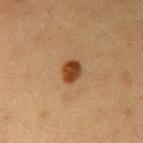Recorded during total-body skin imaging; not selected for excision or biopsy. The recorded lesion diameter is about 2.5 mm. Located on the right upper arm. The total-body-photography lesion software estimated an eccentricity of roughly 0.65 and a symmetry-axis asymmetry near 0.2. The analysis additionally found a mean CIELAB color near L≈34 a*≈20 b*≈32 and a normalized border contrast of about 11.5. It also reported a nevus-likeness score of about 100/100 and a detector confidence of about 100 out of 100 that the crop contains a lesion. The patient is a male about 40 years old. A lesion tile, about 15 mm wide, cut from a 3D total-body photograph. Captured under cross-polarized illumination.